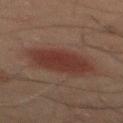Captured during whole-body skin photography for melanoma surveillance; the lesion was not biopsied. From the mid back. A male patient, aged around 45. Measured at roughly 6 mm in maximum diameter. A 15 mm close-up extracted from a 3D total-body photography capture.